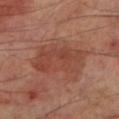Imaged during a routine full-body skin examination; the lesion was not biopsied and no histopathology is available.
The patient is a male aged 68–72.
Captured under cross-polarized illumination.
On the leg.
A 15 mm close-up tile from a total-body photography series done for melanoma screening.
An algorithmic analysis of the crop reported an eccentricity of roughly 0.85 and two-axis asymmetry of about 0.25. And it measured an automated nevus-likeness rating near 5 out of 100 and lesion-presence confidence of about 100/100.
The recorded lesion diameter is about 7 mm.